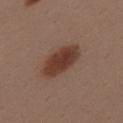Captured during whole-body skin photography for melanoma surveillance; the lesion was not biopsied. The tile uses white-light illumination. A male patient, aged 38 to 42. From the upper back. Approximately 5.5 mm at its widest. A 15 mm crop from a total-body photograph taken for skin-cancer surveillance.